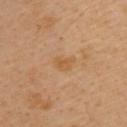Impression:
Captured during whole-body skin photography for melanoma surveillance; the lesion was not biopsied.
Context:
A male subject in their 40s. A 15 mm crop from a total-body photograph taken for skin-cancer surveillance. On the back. Imaged with cross-polarized lighting.